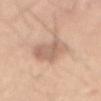A male subject aged approximately 50. The lesion is on the abdomen. This is a white-light tile. A lesion tile, about 15 mm wide, cut from a 3D total-body photograph. Approximately 7 mm at its widest. Automated image analysis of the tile measured an average lesion color of about L≈63 a*≈18 b*≈28 (CIELAB). The software also gave a border-irregularity rating of about 5/10 and radial color variation of about 1.